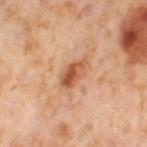patient — female, aged around 55
lesion size — ≈3.5 mm
tile lighting — cross-polarized
imaging modality — ~15 mm crop, total-body skin-cancer survey
body site — the right thigh